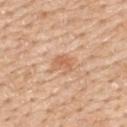Recorded during total-body skin imaging; not selected for excision or biopsy.
The lesion is on the upper back.
The patient is a male aged around 60.
An algorithmic analysis of the crop reported a lesion color around L≈63 a*≈23 b*≈35 in CIELAB, roughly 8 lightness units darker than nearby skin, and a lesion-to-skin contrast of about 6 (normalized; higher = more distinct). And it measured a classifier nevus-likeness of about 0/100 and a lesion-detection confidence of about 100/100.
A close-up tile cropped from a whole-body skin photograph, about 15 mm across.